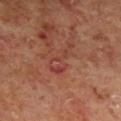The lesion was photographed on a routine skin check and not biopsied; there is no pathology result. The total-body-photography lesion software estimated a lesion area of about 6 mm², an eccentricity of roughly 0.95, and a symmetry-axis asymmetry near 0.65. It also reported a lesion–skin lightness drop of about 6 and a normalized border contrast of about 5. And it measured a border-irregularity index near 8.5/10, a color-variation rating of about 5/10, and radial color variation of about 2. The patient is a male in their 60s. A 15 mm close-up tile from a total-body photography series done for melanoma screening. The lesion is on the right lower leg. This is a cross-polarized tile.